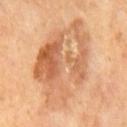Notes:
* workup: total-body-photography surveillance lesion; no biopsy